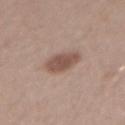notes: total-body-photography surveillance lesion; no biopsy | subject: male, about 30 years old | illumination: white-light illumination | size: ~4 mm (longest diameter) | imaging modality: total-body-photography crop, ~15 mm field of view | automated lesion analysis: an eccentricity of roughly 0.7 and a symmetry-axis asymmetry near 0.15; border irregularity of about 2 on a 0–10 scale, internal color variation of about 2 on a 0–10 scale, and a peripheral color-asymmetry measure near 0.5 | anatomic site: the right upper arm.